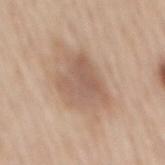| field | value |
|---|---|
| workup | no biopsy performed (imaged during a skin exam) |
| image source | ~15 mm tile from a whole-body skin photo |
| lesion size | ≈5.5 mm |
| patient | male, aged 63 to 67 |
| automated metrics | a lesion color around L≈58 a*≈17 b*≈28 in CIELAB, a lesion–skin lightness drop of about 9, and a normalized lesion–skin contrast near 6; border irregularity of about 3 on a 0–10 scale, a within-lesion color-variation index near 4/10, and a peripheral color-asymmetry measure near 1.5 |
| lighting | white-light |
| anatomic site | the mid back |